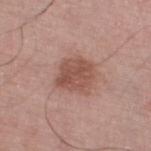{"biopsy_status": "not biopsied; imaged during a skin examination", "patient": {"sex": "male", "age_approx": 70}, "lesion_size": {"long_diameter_mm_approx": 4.0}, "automated_metrics": {"eccentricity": 0.45, "shape_asymmetry": 0.15, "cielab_L": 51, "cielab_a": 22, "cielab_b": 26, "vs_skin_contrast_norm": 7.5, "border_irregularity_0_10": 2.0, "peripheral_color_asymmetry": 1.5}, "image": {"source": "total-body photography crop", "field_of_view_mm": 15}, "site": "right thigh"}